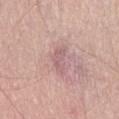Recorded during total-body skin imaging; not selected for excision or biopsy.
From the right thigh.
A male patient about 55 years old.
A lesion tile, about 15 mm wide, cut from a 3D total-body photograph.